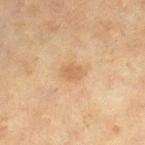Clinical impression: Recorded during total-body skin imaging; not selected for excision or biopsy. Image and clinical context: A 15 mm close-up tile from a total-body photography series done for melanoma screening. The subject is a female roughly 55 years of age. The tile uses cross-polarized illumination. Located on the right lower leg. Longest diameter approximately 3 mm.